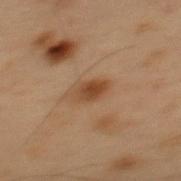Assessment:
This lesion was catalogued during total-body skin photography and was not selected for biopsy.
Context:
On the mid back. The patient is a male aged 53 to 57. This is a cross-polarized tile. A roughly 15 mm field-of-view crop from a total-body skin photograph.